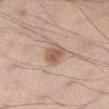The lesion was tiled from a total-body skin photograph and was not biopsied. Automated image analysis of the tile measured a mean CIELAB color near L≈55 a*≈18 b*≈27 and a lesion–skin lightness drop of about 10. A roughly 15 mm field-of-view crop from a total-body skin photograph. This is a cross-polarized tile. Located on the leg. A male subject aged approximately 40.